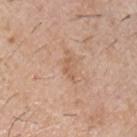Part of a total-body skin-imaging series; this lesion was reviewed on a skin check and was not flagged for biopsy. The total-body-photography lesion software estimated a lesion color around L≈59 a*≈20 b*≈33 in CIELAB, a lesion–skin lightness drop of about 7, and a normalized border contrast of about 5.5. A male subject, approximately 40 years of age. The lesion is on the chest. Captured under white-light illumination. Approximately 2.5 mm at its widest. Cropped from a whole-body photographic skin survey; the tile spans about 15 mm.